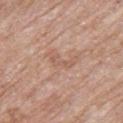biopsy status: no biopsy performed (imaged during a skin exam)
lesion diameter: about 3.5 mm
illumination: white-light illumination
anatomic site: the upper back
image-analysis metrics: roughly 7 lightness units darker than nearby skin and a normalized lesion–skin contrast near 4.5; a nevus-likeness score of about 0/100 and lesion-presence confidence of about 100/100
patient: female, approximately 85 years of age or older
acquisition: 15 mm crop, total-body photography